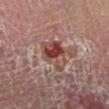Q: How large is the lesion?
A: about 5 mm
Q: How was this image acquired?
A: ~15 mm tile from a whole-body skin photo
Q: Where on the body is the lesion?
A: the left lower leg
Q: What are the patient's age and sex?
A: male, in their mid-50s
Q: What did automated image analysis measure?
A: a border-irregularity rating of about 4.5/10, internal color variation of about 9 on a 0–10 scale, and radial color variation of about 3
Q: How was the tile lit?
A: cross-polarized